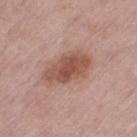biopsy status: imaged on a skin check; not biopsied
diameter: ≈5.5 mm
image-analysis metrics: an area of roughly 14 mm² and a symmetry-axis asymmetry near 0.15; a border-irregularity rating of about 2/10, a color-variation rating of about 4/10, and a peripheral color-asymmetry measure near 1
imaging modality: ~15 mm tile from a whole-body skin photo
site: the leg
patient: female, about 40 years old
tile lighting: white-light